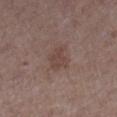lesion size: ~3 mm (longest diameter) | location: the right lower leg | lighting: white-light illumination | automated lesion analysis: a footprint of about 6 mm² and an eccentricity of roughly 0.65; a border-irregularity rating of about 3.5/10, a within-lesion color-variation index near 2/10, and peripheral color asymmetry of about 0.5 | acquisition: ~15 mm crop, total-body skin-cancer survey | patient: female, approximately 40 years of age.